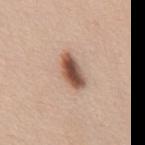Clinical impression: Part of a total-body skin-imaging series; this lesion was reviewed on a skin check and was not flagged for biopsy. Clinical summary: The lesion is located on the abdomen. The patient is a female aged around 45. Cropped from a whole-body photographic skin survey; the tile spans about 15 mm.